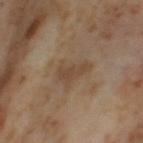Imaged during a routine full-body skin examination; the lesion was not biopsied and no histopathology is available. This is a cross-polarized tile. On the leg. The total-body-photography lesion software estimated a footprint of about 7 mm² and two-axis asymmetry of about 0.4. It also reported a border-irregularity index near 4.5/10, a color-variation rating of about 1.5/10, and peripheral color asymmetry of about 0.5. And it measured an automated nevus-likeness rating near 0 out of 100. A 15 mm close-up extracted from a 3D total-body photography capture. The patient is a female approximately 55 years of age.